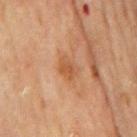{
  "biopsy_status": "not biopsied; imaged during a skin examination",
  "lighting": "cross-polarized",
  "site": "mid back",
  "lesion_size": {
    "long_diameter_mm_approx": 2.5
  },
  "image": {
    "source": "total-body photography crop",
    "field_of_view_mm": 15
  },
  "patient": {
    "sex": "male",
    "age_approx": 70
  }
}